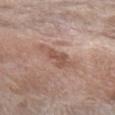This lesion was catalogued during total-body skin photography and was not selected for biopsy.
An algorithmic analysis of the crop reported a footprint of about 7 mm² and an outline eccentricity of about 0.9 (0 = round, 1 = elongated). The analysis additionally found an average lesion color of about L≈54 a*≈19 b*≈27 (CIELAB) and a lesion-to-skin contrast of about 6.5 (normalized; higher = more distinct). It also reported a nevus-likeness score of about 0/100 and lesion-presence confidence of about 100/100.
From the left forearm.
A 15 mm close-up tile from a total-body photography series done for melanoma screening.
The recorded lesion diameter is about 4.5 mm.
Imaged with white-light lighting.
The subject is a female aged approximately 75.